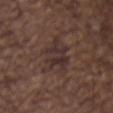Captured during whole-body skin photography for melanoma surveillance; the lesion was not biopsied.
On the chest.
A male subject, aged around 50.
A lesion tile, about 15 mm wide, cut from a 3D total-body photograph.
The lesion-visualizer software estimated a border-irregularity rating of about 7/10, a within-lesion color-variation index near 4/10, and radial color variation of about 1.5. It also reported an automated nevus-likeness rating near 0 out of 100.
The lesion's longest dimension is about 6 mm.
This is a white-light tile.